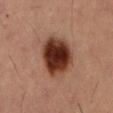No biopsy was performed on this lesion — it was imaged during a full skin examination and was not determined to be concerning. The lesion's longest dimension is about 5 mm. A male patient roughly 55 years of age. A 15 mm close-up tile from a total-body photography series done for melanoma screening. The lesion is on the abdomen.